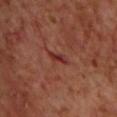Assessment:
No biopsy was performed on this lesion — it was imaged during a full skin examination and was not determined to be concerning.
Acquisition and patient details:
The lesion's longest dimension is about 3 mm. Located on the chest. Automated tile analysis of the lesion measured an area of roughly 3 mm², an outline eccentricity of about 0.9 (0 = round, 1 = elongated), and two-axis asymmetry of about 0.35. The analysis additionally found an average lesion color of about L≈32 a*≈28 b*≈25 (CIELAB), a lesion–skin lightness drop of about 8, and a normalized border contrast of about 7.5. And it measured lesion-presence confidence of about 90/100. The patient is a male about 70 years old. This is a cross-polarized tile. A region of skin cropped from a whole-body photographic capture, roughly 15 mm wide.